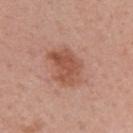biopsy status: imaged on a skin check; not biopsied | lighting: white-light illumination | acquisition: ~15 mm crop, total-body skin-cancer survey | patient: female, approximately 55 years of age | location: the left upper arm.